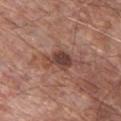{
  "biopsy_status": "not biopsied; imaged during a skin examination",
  "site": "chest",
  "lesion_size": {
    "long_diameter_mm_approx": 4.5
  },
  "lighting": "white-light",
  "image": {
    "source": "total-body photography crop",
    "field_of_view_mm": 15
  },
  "patient": {
    "sex": "male",
    "age_approx": 65
  }
}